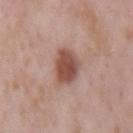| field | value |
|---|---|
| workup | imaged on a skin check; not biopsied |
| location | the mid back |
| acquisition | total-body-photography crop, ~15 mm field of view |
| lesion diameter | about 4.5 mm |
| image-analysis metrics | a lesion area of about 10 mm² and an eccentricity of roughly 0.75; an average lesion color of about L≈50 a*≈22 b*≈26 (CIELAB) and a lesion-to-skin contrast of about 9.5 (normalized; higher = more distinct); a nevus-likeness score of about 95/100 and a lesion-detection confidence of about 100/100 |
| patient | male, roughly 55 years of age |
| tile lighting | white-light |